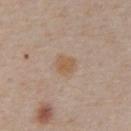Assessment: The lesion was tiled from a total-body skin photograph and was not biopsied. Context: The patient is a male aged 58 to 62. This is a white-light tile. Cropped from a total-body skin-imaging series; the visible field is about 15 mm. The lesion is on the abdomen.